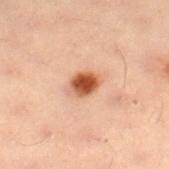The lesion was photographed on a routine skin check and not biopsied; there is no pathology result.